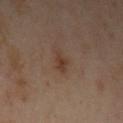* biopsy status · catalogued during a skin exam; not biopsied
* patient · female, roughly 40 years of age
* lighting · cross-polarized illumination
* lesion size · ≈2.5 mm
* imaging modality · ~15 mm crop, total-body skin-cancer survey
* body site · the left upper arm